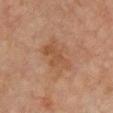{
  "biopsy_status": "not biopsied; imaged during a skin examination",
  "image": {
    "source": "total-body photography crop",
    "field_of_view_mm": 15
  },
  "lesion_size": {
    "long_diameter_mm_approx": 5.0
  },
  "site": "chest",
  "patient": {
    "sex": "female",
    "age_approx": 70
  }
}